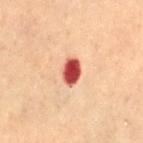Captured during whole-body skin photography for melanoma surveillance; the lesion was not biopsied.
Automated tile analysis of the lesion measured a classifier nevus-likeness of about 0/100 and a lesion-detection confidence of about 100/100.
On the mid back.
The subject is a female aged around 80.
A lesion tile, about 15 mm wide, cut from a 3D total-body photograph.